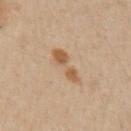Captured during whole-body skin photography for melanoma surveillance; the lesion was not biopsied.